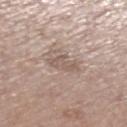- biopsy status: no biopsy performed (imaged during a skin exam)
- diameter: about 3.5 mm
- TBP lesion metrics: a mean CIELAB color near L≈56 a*≈15 b*≈23, a lesion–skin lightness drop of about 9, and a normalized lesion–skin contrast near 6; border irregularity of about 4.5 on a 0–10 scale, internal color variation of about 1.5 on a 0–10 scale, and peripheral color asymmetry of about 0.5
- image: 15 mm crop, total-body photography
- tile lighting: white-light illumination
- subject: male, roughly 65 years of age
- anatomic site: the left lower leg